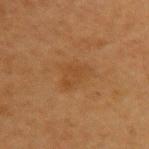| field | value |
|---|---|
| follow-up | catalogued during a skin exam; not biopsied |
| tile lighting | cross-polarized illumination |
| body site | the left upper arm |
| imaging modality | ~15 mm crop, total-body skin-cancer survey |
| subject | female, in their 40s |